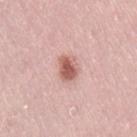Background: About 3 mm across. Cropped from a whole-body photographic skin survey; the tile spans about 15 mm. The lesion is located on the lower back. A female subject approximately 40 years of age. Automated image analysis of the tile measured an eccentricity of roughly 0.65. The software also gave an automated nevus-likeness rating near 90 out of 100 and a lesion-detection confidence of about 100/100.